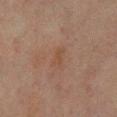The lesion was photographed on a routine skin check and not biopsied; there is no pathology result. The patient is a female aged 53 to 57. This image is a 15 mm lesion crop taken from a total-body photograph. From the leg.